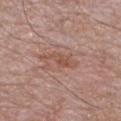The lesion was tiled from a total-body skin photograph and was not biopsied. Longest diameter approximately 4.5 mm. Automated tile analysis of the lesion measured a mean CIELAB color near L≈52 a*≈22 b*≈27, a lesion–skin lightness drop of about 8, and a normalized lesion–skin contrast near 6.5. The analysis additionally found a border-irregularity rating of about 4.5/10, internal color variation of about 2.5 on a 0–10 scale, and peripheral color asymmetry of about 0.5. The analysis additionally found an automated nevus-likeness rating near 0 out of 100 and a lesion-detection confidence of about 100/100. Imaged with white-light lighting. A close-up tile cropped from a whole-body skin photograph, about 15 mm across. On the chest. A male subject in their 70s.